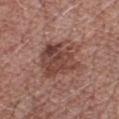The lesion was tiled from a total-body skin photograph and was not biopsied.
A lesion tile, about 15 mm wide, cut from a 3D total-body photograph.
The lesion is on the chest.
Automated image analysis of the tile measured a within-lesion color-variation index near 6.5/10 and peripheral color asymmetry of about 2.5.
This is a white-light tile.
A male patient aged approximately 65.